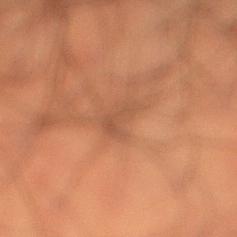Background: This image is a 15 mm lesion crop taken from a total-body photograph. A male patient, aged 48–52. The tile uses cross-polarized illumination. The recorded lesion diameter is about 3 mm. Automated image analysis of the tile measured an area of roughly 4 mm², an eccentricity of roughly 0.8, and two-axis asymmetry of about 0.5. And it measured a mean CIELAB color near L≈40 a*≈19 b*≈28. The analysis additionally found an automated nevus-likeness rating near 0 out of 100 and a lesion-detection confidence of about 60/100. The lesion is located on the leg.